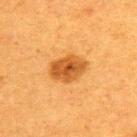The lesion was photographed on a routine skin check and not biopsied; there is no pathology result. The subject is a female roughly 40 years of age. The lesion is located on the back. A 15 mm close-up extracted from a 3D total-body photography capture. Imaged with cross-polarized lighting. About 4.5 mm across.